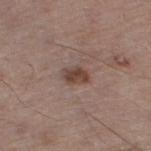Assessment:
Part of a total-body skin-imaging series; this lesion was reviewed on a skin check and was not flagged for biopsy.
Context:
The subject is a male aged 63–67. Captured under white-light illumination. The lesion is located on the left thigh. A region of skin cropped from a whole-body photographic capture, roughly 15 mm wide. The total-body-photography lesion software estimated a lesion area of about 4.5 mm² and a shape eccentricity near 0.7. It also reported about 11 CIELAB-L* units darker than the surrounding skin and a normalized border contrast of about 8.5.